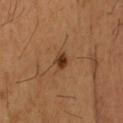{
  "biopsy_status": "not biopsied; imaged during a skin examination",
  "site": "head or neck",
  "patient": {
    "sex": "male",
    "age_approx": 45
  },
  "image": {
    "source": "total-body photography crop",
    "field_of_view_mm": 15
  },
  "lighting": "cross-polarized",
  "automated_metrics": {
    "border_irregularity_0_10": 2.0,
    "peripheral_color_asymmetry": 1.0,
    "nevus_likeness_0_100": 95,
    "lesion_detection_confidence_0_100": 100
  },
  "lesion_size": {
    "long_diameter_mm_approx": 2.0
  }
}